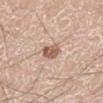* image · total-body-photography crop, ~15 mm field of view
* patient · male, in their 50s
* lesion size · ≈3 mm
* lighting · white-light
* location · the left lower leg
* automated lesion analysis · a border-irregularity index near 2.5/10, a color-variation rating of about 3/10, and a peripheral color-asymmetry measure near 1; an automated nevus-likeness rating near 90 out of 100